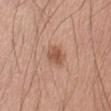A male subject in their mid- to late 50s. This is a white-light tile. About 2.5 mm across. The lesion-visualizer software estimated a mean CIELAB color near L≈54 a*≈23 b*≈31 and a normalized border contrast of about 7.5. The analysis additionally found a border-irregularity index near 3/10 and a peripheral color-asymmetry measure near 1. It also reported a nevus-likeness score of about 85/100 and a detector confidence of about 100 out of 100 that the crop contains a lesion. A 15 mm close-up tile from a total-body photography series done for melanoma screening.